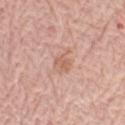Clinical impression: Recorded during total-body skin imaging; not selected for excision or biopsy. Image and clinical context: A lesion tile, about 15 mm wide, cut from a 3D total-body photograph. An algorithmic analysis of the crop reported a footprint of about 5 mm², a shape eccentricity near 0.65, and two-axis asymmetry of about 0.3. The analysis additionally found lesion-presence confidence of about 100/100. The lesion is located on the left forearm. The subject is a female in their 70s. The lesion's longest dimension is about 3 mm. The tile uses white-light illumination.